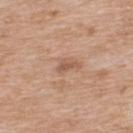No biopsy was performed on this lesion — it was imaged during a full skin examination and was not determined to be concerning.
A lesion tile, about 15 mm wide, cut from a 3D total-body photograph.
A male patient in their 50s.
This is a white-light tile.
Longest diameter approximately 2.5 mm.
From the upper back.